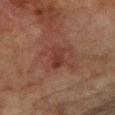workup = catalogued during a skin exam; not biopsied | TBP lesion metrics = a mean CIELAB color near L≈31 a*≈22 b*≈23, a lesion–skin lightness drop of about 7, and a normalized lesion–skin contrast near 6.5; a border-irregularity rating of about 2.5/10 and a within-lesion color-variation index near 3/10; a nevus-likeness score of about 0/100 and lesion-presence confidence of about 100/100 | image source = 15 mm crop, total-body photography | lesion size = ≈3 mm | subject = female, aged approximately 80 | body site = the left forearm | tile lighting = cross-polarized illumination.